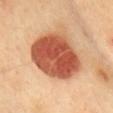Q: Was this lesion biopsied?
A: catalogued during a skin exam; not biopsied